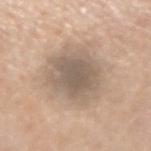Imaged during a routine full-body skin examination; the lesion was not biopsied and no histopathology is available.
The total-body-photography lesion software estimated a lesion area of about 19 mm² and two-axis asymmetry of about 0.2. The software also gave a mean CIELAB color near L≈57 a*≈12 b*≈25. It also reported a border-irregularity rating of about 2.5/10, a within-lesion color-variation index near 3/10, and radial color variation of about 1. It also reported a nevus-likeness score of about 15/100 and a detector confidence of about 90 out of 100 that the crop contains a lesion.
The recorded lesion diameter is about 5.5 mm.
A female subject aged around 45.
From the arm.
Captured under white-light illumination.
A roughly 15 mm field-of-view crop from a total-body skin photograph.